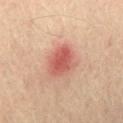Imaged during a routine full-body skin examination; the lesion was not biopsied and no histopathology is available. The lesion is located on the lower back. A male subject in their mid- to late 60s. Automated image analysis of the tile measured an area of roughly 11 mm², an eccentricity of roughly 0.6, and two-axis asymmetry of about 0.25. The analysis additionally found a mean CIELAB color near L≈51 a*≈26 b*≈26, about 11 CIELAB-L* units darker than the surrounding skin, and a normalized border contrast of about 7.5. The analysis additionally found lesion-presence confidence of about 100/100. A close-up tile cropped from a whole-body skin photograph, about 15 mm across. Imaged with cross-polarized lighting. The recorded lesion diameter is about 4 mm.